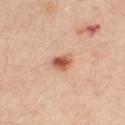The lesion was tiled from a total-body skin photograph and was not biopsied. A female patient in their 40s. The tile uses cross-polarized illumination. Located on the right leg. Measured at roughly 3 mm in maximum diameter. A 15 mm crop from a total-body photograph taken for skin-cancer surveillance. Automated image analysis of the tile measured a lesion area of about 5 mm², a shape eccentricity near 0.6, and two-axis asymmetry of about 0.25. It also reported lesion-presence confidence of about 100/100.